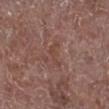Impression:
Recorded during total-body skin imaging; not selected for excision or biopsy.
Context:
The lesion-visualizer software estimated an area of roughly 3 mm² and a shape eccentricity near 0.9. It also reported a border-irregularity index near 7/10, internal color variation of about 0 on a 0–10 scale, and radial color variation of about 0. A 15 mm close-up extracted from a 3D total-body photography capture. A male patient approximately 70 years of age. On the right lower leg. The tile uses white-light illumination.